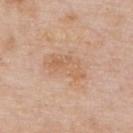Assessment:
This lesion was catalogued during total-body skin photography and was not selected for biopsy.
Clinical summary:
Approximately 4.5 mm at its widest. A lesion tile, about 15 mm wide, cut from a 3D total-body photograph. Imaged with white-light lighting. A male patient in their mid-70s. The lesion is located on the upper back.